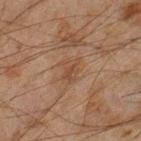Imaged during a routine full-body skin examination; the lesion was not biopsied and no histopathology is available. The lesion is located on the right lower leg. This is a cross-polarized tile. About 3 mm across. The subject is a male about 45 years old. Automated tile analysis of the lesion measured a footprint of about 4.5 mm², an outline eccentricity of about 0.75 (0 = round, 1 = elongated), and a shape-asymmetry score of about 0.2 (0 = symmetric). The analysis additionally found a mean CIELAB color near L≈37 a*≈16 b*≈26 and roughly 5 lightness units darker than nearby skin. Cropped from a total-body skin-imaging series; the visible field is about 15 mm.